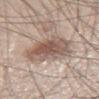| field | value |
|---|---|
| workup | total-body-photography surveillance lesion; no biopsy |
| automated metrics | a mean CIELAB color near L≈55 a*≈16 b*≈24, a lesion–skin lightness drop of about 13, and a normalized lesion–skin contrast near 8.5 |
| acquisition | 15 mm crop, total-body photography |
| patient | male, in their 60s |
| anatomic site | the leg |